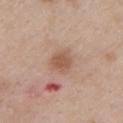Imaged during a routine full-body skin examination; the lesion was not biopsied and no histopathology is available.